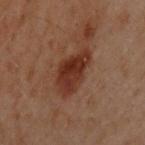Clinical impression: Imaged during a routine full-body skin examination; the lesion was not biopsied and no histopathology is available. Background: The lesion-visualizer software estimated an area of roughly 12 mm², an eccentricity of roughly 0.85, and a shape-asymmetry score of about 0.25 (0 = symmetric). It also reported an automated nevus-likeness rating near 80 out of 100 and a lesion-detection confidence of about 100/100. The tile uses cross-polarized illumination. Longest diameter approximately 5.5 mm. On the upper back. A male patient, roughly 50 years of age. This image is a 15 mm lesion crop taken from a total-body photograph.